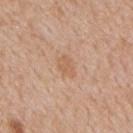Part of a total-body skin-imaging series; this lesion was reviewed on a skin check and was not flagged for biopsy. Imaged with white-light lighting. The lesion is on the mid back. Longest diameter approximately 3 mm. A close-up tile cropped from a whole-body skin photograph, about 15 mm across. A male patient, in their mid- to late 60s.